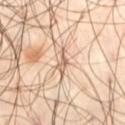Q: Lesion location?
A: the left thigh
Q: Illumination type?
A: cross-polarized illumination
Q: What are the patient's age and sex?
A: male, roughly 40 years of age
Q: Automated lesion metrics?
A: a lesion area of about 3 mm² and a shape-asymmetry score of about 0.45 (0 = symmetric); an automated nevus-likeness rating near 0 out of 100
Q: What is the imaging modality?
A: 15 mm crop, total-body photography
Q: How large is the lesion?
A: about 3 mm